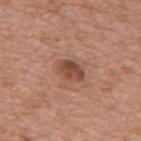Clinical impression: The lesion was photographed on a routine skin check and not biopsied; there is no pathology result. Acquisition and patient details: Cropped from a total-body skin-imaging series; the visible field is about 15 mm. A female subject, in their mid-70s. The lesion is located on the mid back. The lesion-visualizer software estimated a border-irregularity rating of about 1.5/10, internal color variation of about 4 on a 0–10 scale, and radial color variation of about 1.5. And it measured lesion-presence confidence of about 100/100. Captured under white-light illumination.